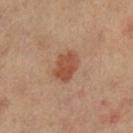Assessment: Imaged during a routine full-body skin examination; the lesion was not biopsied and no histopathology is available. Acquisition and patient details: About 3.5 mm across. This is a cross-polarized tile. From the right leg. A female patient aged 63 to 67. A 15 mm close-up tile from a total-body photography series done for melanoma screening.